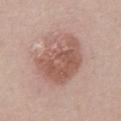Captured under white-light illumination.
A roughly 15 mm field-of-view crop from a total-body skin photograph.
The patient is a male in their mid-50s.
The lesion is on the abdomen.
An algorithmic analysis of the crop reported a border-irregularity index near 2/10. It also reported a classifier nevus-likeness of about 30/100 and a detector confidence of about 100 out of 100 that the crop contains a lesion.